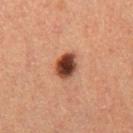Q: What are the patient's age and sex?
A: female, aged 38 to 42
Q: How was the tile lit?
A: cross-polarized
Q: Lesion size?
A: ≈3 mm
Q: How was this image acquired?
A: ~15 mm tile from a whole-body skin photo
Q: Automated lesion metrics?
A: a mean CIELAB color near L≈35 a*≈22 b*≈26, roughly 18 lightness units darker than nearby skin, and a normalized border contrast of about 14.5; border irregularity of about 1.5 on a 0–10 scale, a within-lesion color-variation index near 5/10, and peripheral color asymmetry of about 1.5
Q: Lesion location?
A: the leg